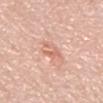The lesion was photographed on a routine skin check and not biopsied; there is no pathology result. A region of skin cropped from a whole-body photographic capture, roughly 15 mm wide. The subject is a male about 80 years old. On the abdomen. Captured under white-light illumination.